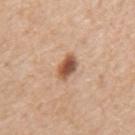The lesion was tiled from a total-body skin photograph and was not biopsied. About 3 mm across. The lesion is located on the mid back. A male patient, aged 68 to 72. A roughly 15 mm field-of-view crop from a total-body skin photograph. An algorithmic analysis of the crop reported an area of roughly 5 mm² and a symmetry-axis asymmetry near 0.2. The analysis additionally found border irregularity of about 2 on a 0–10 scale and a color-variation rating of about 5.5/10. And it measured a detector confidence of about 100 out of 100 that the crop contains a lesion. The tile uses white-light illumination.